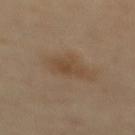The patient is a female approximately 70 years of age. A 15 mm close-up tile from a total-body photography series done for melanoma screening. The lesion is located on the mid back.